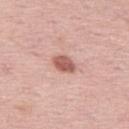Assessment:
Captured during whole-body skin photography for melanoma surveillance; the lesion was not biopsied.
Acquisition and patient details:
The subject is a female in their mid- to late 60s. This is a white-light tile. Longest diameter approximately 3 mm. The lesion is on the left thigh. A 15 mm close-up tile from a total-body photography series done for melanoma screening. The lesion-visualizer software estimated a lesion area of about 5 mm², a shape eccentricity near 0.75, and two-axis asymmetry of about 0.15. The software also gave an average lesion color of about L≈58 a*≈25 b*≈26 (CIELAB), about 14 CIELAB-L* units darker than the surrounding skin, and a normalized border contrast of about 9. And it measured a border-irregularity rating of about 1/10, internal color variation of about 3 on a 0–10 scale, and peripheral color asymmetry of about 1. And it measured a classifier nevus-likeness of about 90/100 and a lesion-detection confidence of about 100/100.